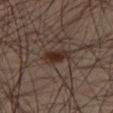Background: The recorded lesion diameter is about 3.5 mm. A region of skin cropped from a whole-body photographic capture, roughly 15 mm wide. Automated image analysis of the tile measured an area of roughly 5.5 mm², an outline eccentricity of about 0.85 (0 = round, 1 = elongated), and two-axis asymmetry of about 0.25. A male patient about 45 years old. The lesion is on the right thigh.